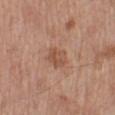biopsy status = imaged on a skin check; not biopsied
image = ~15 mm crop, total-body skin-cancer survey
location = the left lower leg
tile lighting = white-light illumination
lesion diameter = about 3.5 mm
subject = male, approximately 70 years of age